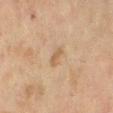• biopsy status — imaged on a skin check; not biopsied
• body site — the right lower leg
• lighting — cross-polarized illumination
• image — ~15 mm tile from a whole-body skin photo
• TBP lesion metrics — a mean CIELAB color near L≈52 a*≈15 b*≈31, about 7 CIELAB-L* units darker than the surrounding skin, and a lesion-to-skin contrast of about 5.5 (normalized; higher = more distinct); border irregularity of about 3 on a 0–10 scale
• patient — female, approximately 55 years of age
• size — ~2.5 mm (longest diameter)